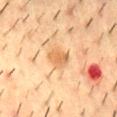No biopsy was performed on this lesion — it was imaged during a full skin examination and was not determined to be concerning.
The recorded lesion diameter is about 2.5 mm.
A male subject approximately 55 years of age.
A 15 mm crop from a total-body photograph taken for skin-cancer surveillance.
The lesion is on the back.
Imaged with cross-polarized lighting.